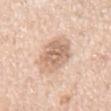| key | value |
|---|---|
| image | ~15 mm tile from a whole-body skin photo |
| location | the chest |
| patient | male, aged around 60 |
| illumination | white-light |
| lesion diameter | ≈5.5 mm |